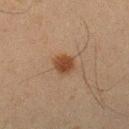The lesion was photographed on a routine skin check and not biopsied; there is no pathology result.
Imaged with cross-polarized lighting.
Approximately 2.5 mm at its widest.
On the left forearm.
A 15 mm close-up tile from a total-body photography series done for melanoma screening.
The patient is a male about 35 years old.